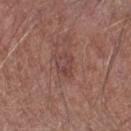follow-up = no biopsy performed (imaged during a skin exam)
diameter = ≈3 mm
image source = 15 mm crop, total-body photography
site = the left forearm
tile lighting = white-light illumination
patient = male, in their 70s